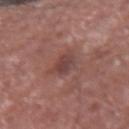From the right forearm. This image is a 15 mm lesion crop taken from a total-body photograph. A male patient, aged 63–67.